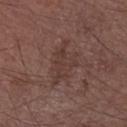Q: Is there a histopathology result?
A: catalogued during a skin exam; not biopsied
Q: Illumination type?
A: white-light
Q: Patient demographics?
A: male, aged 73–77
Q: What is the lesion's diameter?
A: ≈5.5 mm
Q: What is the imaging modality?
A: 15 mm crop, total-body photography
Q: Lesion location?
A: the right forearm